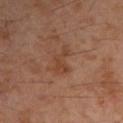* workup: no biopsy performed (imaged during a skin exam)
* patient: male, roughly 55 years of age
* lighting: cross-polarized illumination
* anatomic site: the left upper arm
* imaging modality: ~15 mm tile from a whole-body skin photo